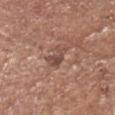The lesion was photographed on a routine skin check and not biopsied; there is no pathology result.
A male subject, in their 70s.
A 15 mm close-up tile from a total-body photography series done for melanoma screening.
The lesion is located on the head or neck.